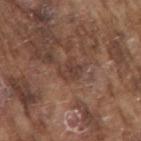Notes:
* notes: catalogued during a skin exam; not biopsied
* body site: the right upper arm
* patient: male, approximately 75 years of age
* image-analysis metrics: a lesion area of about 5 mm² and two-axis asymmetry of about 0.3; an average lesion color of about L≈40 a*≈18 b*≈25 (CIELAB), about 7 CIELAB-L* units darker than the surrounding skin, and a lesion-to-skin contrast of about 6 (normalized; higher = more distinct); a detector confidence of about 80 out of 100 that the crop contains a lesion
* lesion size: ~3 mm (longest diameter)
* illumination: white-light
* imaging modality: ~15 mm crop, total-body skin-cancer survey